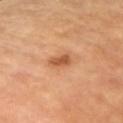Assessment: Recorded during total-body skin imaging; not selected for excision or biopsy. Context: A female subject aged approximately 60. A 15 mm close-up extracted from a 3D total-body photography capture. On the left arm.